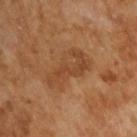<lesion>
  <biopsy_status>not biopsied; imaged during a skin examination</biopsy_status>
  <lighting>cross-polarized</lighting>
  <automated_metrics>
    <eccentricity>0.85</eccentricity>
    <shape_asymmetry>0.35</shape_asymmetry>
    <cielab_L>44</cielab_L>
    <cielab_a>22</cielab_a>
    <cielab_b>34</cielab_b>
    <vs_skin_contrast_norm>5.5</vs_skin_contrast_norm>
    <nevus_likeness_0_100>0</nevus_likeness_0_100>
    <lesion_detection_confidence_0_100>100</lesion_detection_confidence_0_100>
  </automated_metrics>
  <patient>
    <sex>male</sex>
    <age_approx>65</age_approx>
  </patient>
  <lesion_size>
    <long_diameter_mm_approx>6.0</long_diameter_mm_approx>
  </lesion_size>
  <image>
    <source>total-body photography crop</source>
    <field_of_view_mm>15</field_of_view_mm>
  </image>
</lesion>